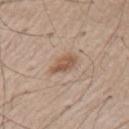Q: Was a biopsy performed?
A: no biopsy performed (imaged during a skin exam)
Q: Lesion size?
A: about 3.5 mm
Q: Patient demographics?
A: male, in their mid-50s
Q: How was the tile lit?
A: white-light illumination
Q: What is the imaging modality?
A: total-body-photography crop, ~15 mm field of view